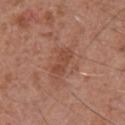workup: total-body-photography surveillance lesion; no biopsy | lighting: white-light illumination | anatomic site: the front of the torso | size: ~3.5 mm (longest diameter) | image source: total-body-photography crop, ~15 mm field of view | automated metrics: an area of roughly 5.5 mm², an eccentricity of roughly 0.9, and a shape-asymmetry score of about 0.2 (0 = symmetric); a nevus-likeness score of about 0/100 and a detector confidence of about 100 out of 100 that the crop contains a lesion | patient: male, approximately 65 years of age.